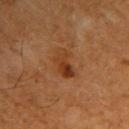Longest diameter approximately 4 mm. A lesion tile, about 15 mm wide, cut from a 3D total-body photograph. Captured under cross-polarized illumination. A male patient, aged approximately 60. The lesion is located on the upper back. An algorithmic analysis of the crop reported a border-irregularity index near 3.5/10, a color-variation rating of about 7.5/10, and radial color variation of about 3.